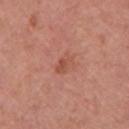<lesion>
  <automated_metrics>
    <cielab_L>52</cielab_L>
    <cielab_a>27</cielab_a>
    <cielab_b>30</cielab_b>
    <vs_skin_darker_L>7.0</vs_skin_darker_L>
    <color_variation_0_10>3.0</color_variation_0_10>
    <peripheral_color_asymmetry>1.0</peripheral_color_asymmetry>
    <nevus_likeness_0_100>5</nevus_likeness_0_100>
    <lesion_detection_confidence_0_100>100</lesion_detection_confidence_0_100>
  </automated_metrics>
  <lighting>white-light</lighting>
  <site>right upper arm</site>
  <patient>
    <sex>female</sex>
    <age_approx>65</age_approx>
  </patient>
  <lesion_size>
    <long_diameter_mm_approx>2.5</long_diameter_mm_approx>
  </lesion_size>
  <image>
    <source>total-body photography crop</source>
    <field_of_view_mm>15</field_of_view_mm>
  </image>
</lesion>